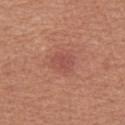Clinical impression: The lesion was photographed on a routine skin check and not biopsied; there is no pathology result. Context: A female subject roughly 60 years of age. The lesion is located on the left thigh. A roughly 15 mm field-of-view crop from a total-body skin photograph.